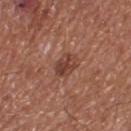<tbp_lesion>
<biopsy_status>not biopsied; imaged during a skin examination</biopsy_status>
<patient>
  <sex>male</sex>
  <age_approx>75</age_approx>
</patient>
<image>
  <source>total-body photography crop</source>
  <field_of_view_mm>15</field_of_view_mm>
</image>
<site>right thigh</site>
<lighting>white-light</lighting>
<lesion_size>
  <long_diameter_mm_approx>3.0</long_diameter_mm_approx>
</lesion_size>
</tbp_lesion>